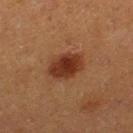Impression: The lesion was tiled from a total-body skin photograph and was not biopsied. Context: The patient is a female aged approximately 40. The lesion's longest dimension is about 4 mm. A close-up tile cropped from a whole-body skin photograph, about 15 mm across. From the left thigh. The tile uses cross-polarized illumination.